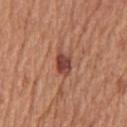Q: Is there a histopathology result?
A: total-body-photography surveillance lesion; no biopsy